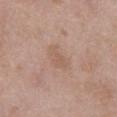Q: Was this lesion biopsied?
A: catalogued during a skin exam; not biopsied